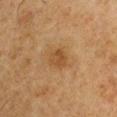biopsy_status: not biopsied; imaged during a skin examination
lesion_size:
  long_diameter_mm_approx: 2.5
patient:
  sex: male
  age_approx: 65
site: arm
lighting: cross-polarized
image:
  source: total-body photography crop
  field_of_view_mm: 15